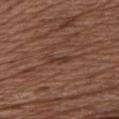• workup — imaged on a skin check; not biopsied
• tile lighting — white-light illumination
• image source — ~15 mm tile from a whole-body skin photo
• body site — the chest
• subject — female, aged 73–77